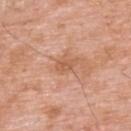The lesion is on the upper back.
The lesion's longest dimension is about 2.5 mm.
Cropped from a whole-body photographic skin survey; the tile spans about 15 mm.
The subject is a male roughly 60 years of age.
Imaged with white-light lighting.